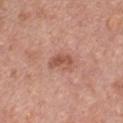No biopsy was performed on this lesion — it was imaged during a full skin examination and was not determined to be concerning.
The lesion is on the front of the torso.
Cropped from a whole-body photographic skin survey; the tile spans about 15 mm.
Imaged with white-light lighting.
The lesion's longest dimension is about 3 mm.
A male patient, aged 58–62.